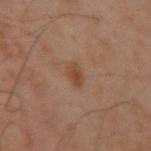- notes · imaged on a skin check; not biopsied
- imaging modality · ~15 mm tile from a whole-body skin photo
- subject · male, aged 48 to 52
- TBP lesion metrics · lesion-presence confidence of about 100/100
- illumination · cross-polarized illumination
- diameter · about 3 mm
- body site · the left arm